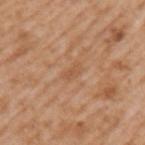No biopsy was performed on this lesion — it was imaged during a full skin examination and was not determined to be concerning.
Automated tile analysis of the lesion measured an area of roughly 3 mm². It also reported an average lesion color of about L≈55 a*≈21 b*≈35 (CIELAB), roughly 6 lightness units darker than nearby skin, and a lesion-to-skin contrast of about 4.5 (normalized; higher = more distinct).
The patient is a male in their mid- to late 60s.
Captured under white-light illumination.
Longest diameter approximately 2.5 mm.
The lesion is located on the left upper arm.
A region of skin cropped from a whole-body photographic capture, roughly 15 mm wide.